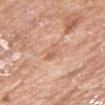Clinical impression:
Captured during whole-body skin photography for melanoma surveillance; the lesion was not biopsied.
Clinical summary:
A close-up tile cropped from a whole-body skin photograph, about 15 mm across. The lesion is on the arm. This is a white-light tile. A female subject, aged 73 to 77. Longest diameter approximately 2.5 mm.